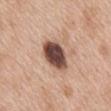The total-body-photography lesion software estimated a lesion color around L≈47 a*≈20 b*≈24 in CIELAB and a normalized border contrast of about 14.5. This is a white-light tile. The recorded lesion diameter is about 5 mm. From the mid back. The subject is a female aged around 40. A region of skin cropped from a whole-body photographic capture, roughly 15 mm wide.